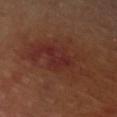Assessment: Captured during whole-body skin photography for melanoma surveillance; the lesion was not biopsied. Acquisition and patient details: The subject is a male aged approximately 65. A roughly 15 mm field-of-view crop from a total-body skin photograph. The lesion is on the right upper arm.